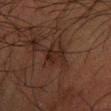follow-up=total-body-photography surveillance lesion; no biopsy
illumination=cross-polarized illumination
subject=male, about 60 years old
body site=the left forearm
lesion diameter=≈3.5 mm
image source=15 mm crop, total-body photography